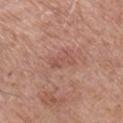A male patient, roughly 75 years of age.
A lesion tile, about 15 mm wide, cut from a 3D total-body photograph.
On the right lower leg.
Captured under white-light illumination.
Measured at roughly 3 mm in maximum diameter.